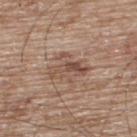follow-up = imaged on a skin check; not biopsied | automated lesion analysis = a border-irregularity index near 5.5/10, a color-variation rating of about 7.5/10, and radial color variation of about 3; a classifier nevus-likeness of about 10/100 and lesion-presence confidence of about 100/100 | image source = ~15 mm tile from a whole-body skin photo | size = about 4.5 mm | lighting = white-light illumination | subject = male, aged approximately 65 | location = the upper back.